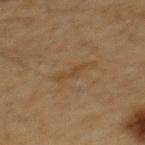Context: A close-up tile cropped from a whole-body skin photograph, about 15 mm across. A male subject approximately 60 years of age. On the mid back.